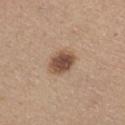A female subject aged around 65. On the chest. Automated tile analysis of the lesion measured a border-irregularity rating of about 1/10 and internal color variation of about 4 on a 0–10 scale. The software also gave an automated nevus-likeness rating near 95 out of 100 and a detector confidence of about 100 out of 100 that the crop contains a lesion. A 15 mm close-up extracted from a 3D total-body photography capture.